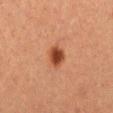A female patient, aged around 40. Cropped from a whole-body photographic skin survey; the tile spans about 15 mm. On the abdomen. The recorded lesion diameter is about 2.5 mm. The total-body-photography lesion software estimated a lesion area of about 5 mm² and an eccentricity of roughly 0.45. It also reported border irregularity of about 1.5 on a 0–10 scale and a color-variation rating of about 2.5/10. And it measured a nevus-likeness score of about 100/100 and a detector confidence of about 100 out of 100 that the crop contains a lesion.